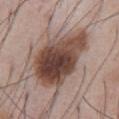A close-up tile cropped from a whole-body skin photograph, about 15 mm across.
A male subject aged approximately 55.
The lesion-visualizer software estimated a border-irregularity rating of about 2.5/10, a within-lesion color-variation index near 8.5/10, and radial color variation of about 2.5.
Captured under white-light illumination.
Longest diameter approximately 8 mm.
Located on the front of the torso.